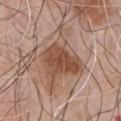Imaged during a routine full-body skin examination; the lesion was not biopsied and no histopathology is available.
On the chest.
A male subject, aged 68 to 72.
Cropped from a whole-body photographic skin survey; the tile spans about 15 mm.